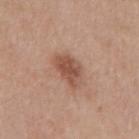Recorded during total-body skin imaging; not selected for excision or biopsy. This is a white-light tile. Approximately 4.5 mm at its widest. A female patient, aged approximately 40. A 15 mm crop from a total-body photograph taken for skin-cancer surveillance. Located on the back. Automated tile analysis of the lesion measured about 11 CIELAB-L* units darker than the surrounding skin. It also reported an automated nevus-likeness rating near 65 out of 100.